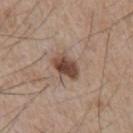biopsy status: catalogued during a skin exam; not biopsied | body site: the chest | size: about 3.5 mm | tile lighting: white-light illumination | image source: ~15 mm crop, total-body skin-cancer survey | automated metrics: a mean CIELAB color near L≈46 a*≈18 b*≈25, roughly 14 lightness units darker than nearby skin, and a lesion-to-skin contrast of about 10.5 (normalized; higher = more distinct); a nevus-likeness score of about 90/100 and a lesion-detection confidence of about 100/100 | subject: male, aged 73 to 77.